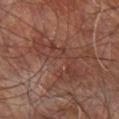Q: Was a biopsy performed?
A: imaged on a skin check; not biopsied
Q: How was this image acquired?
A: total-body-photography crop, ~15 mm field of view
Q: What is the anatomic site?
A: the leg
Q: What are the patient's age and sex?
A: male, about 60 years old
Q: What is the lesion's diameter?
A: ≈7.5 mm